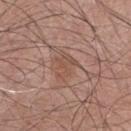Assessment:
Recorded during total-body skin imaging; not selected for excision or biopsy.
Image and clinical context:
A 15 mm crop from a total-body photograph taken for skin-cancer surveillance. The lesion is on the chest. Automated image analysis of the tile measured a mean CIELAB color near L≈50 a*≈20 b*≈27, roughly 6 lightness units darker than nearby skin, and a lesion-to-skin contrast of about 5 (normalized; higher = more distinct). And it measured peripheral color asymmetry of about 0.5. It also reported a classifier nevus-likeness of about 0/100 and a detector confidence of about 95 out of 100 that the crop contains a lesion. Imaged with white-light lighting. A male subject aged 53 to 57.